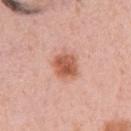workup: catalogued during a skin exam; not biopsied | anatomic site: the arm | patient: female, in their mid- to late 30s | tile lighting: white-light illumination | lesion size: ~3 mm (longest diameter) | automated lesion analysis: a mean CIELAB color near L≈57 a*≈27 b*≈33, about 13 CIELAB-L* units darker than the surrounding skin, and a normalized border contrast of about 9.5; a border-irregularity index near 1.5/10 and a within-lesion color-variation index near 4.5/10; a classifier nevus-likeness of about 100/100 and a detector confidence of about 100 out of 100 that the crop contains a lesion | image source: ~15 mm crop, total-body skin-cancer survey.